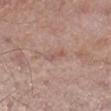| key | value |
|---|---|
| image source | ~15 mm tile from a whole-body skin photo |
| patient | male, about 55 years old |
| illumination | white-light illumination |
| automated metrics | a mean CIELAB color near L≈55 a*≈20 b*≈24, roughly 7 lightness units darker than nearby skin, and a normalized border contrast of about 5 |
| diameter | ≈2.5 mm |
| anatomic site | the right lower leg |